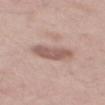Captured during whole-body skin photography for melanoma surveillance; the lesion was not biopsied.
A close-up tile cropped from a whole-body skin photograph, about 15 mm across.
A male patient, in their mid- to late 40s.
Located on the mid back.